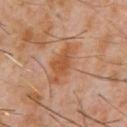Clinical impression: Recorded during total-body skin imaging; not selected for excision or biopsy. Clinical summary: A 15 mm close-up extracted from a 3D total-body photography capture. Captured under cross-polarized illumination. A male patient, aged around 60. From the chest.